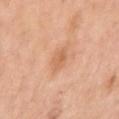Assessment: The lesion was tiled from a total-body skin photograph and was not biopsied. Context: The recorded lesion diameter is about 2.5 mm. Located on the right upper arm. Captured under white-light illumination. A 15 mm close-up tile from a total-body photography series done for melanoma screening. Automated image analysis of the tile measured a shape eccentricity near 0.5 and a symmetry-axis asymmetry near 0.15. The analysis additionally found a mean CIELAB color near L≈64 a*≈23 b*≈37 and a normalized lesion–skin contrast near 5.5. The analysis additionally found an automated nevus-likeness rating near 0 out of 100 and a lesion-detection confidence of about 100/100. The subject is a female about 65 years old.